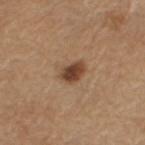follow-up: catalogued during a skin exam; not biopsied.